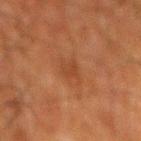Impression:
The lesion was photographed on a routine skin check and not biopsied; there is no pathology result.
Context:
The tile uses cross-polarized illumination. About 2.5 mm across. Cropped from a whole-body photographic skin survey; the tile spans about 15 mm. A male subject, aged 78 to 82. On the mid back.